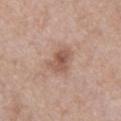Assessment: Recorded during total-body skin imaging; not selected for excision or biopsy. Clinical summary: A region of skin cropped from a whole-body photographic capture, roughly 15 mm wide. On the chest. The lesion's longest dimension is about 3.5 mm. Captured under white-light illumination. A female patient, aged 38–42.